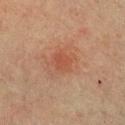{"biopsy_status": "not biopsied; imaged during a skin examination", "patient": {"sex": "female", "age_approx": 55}, "lighting": "cross-polarized", "image": {"source": "total-body photography crop", "field_of_view_mm": 15}, "automated_metrics": {"cielab_L": 40, "cielab_a": 22, "cielab_b": 27, "vs_skin_darker_L": 5.0, "vs_skin_contrast_norm": 5.0, "border_irregularity_0_10": 2.0, "nevus_likeness_0_100": 5, "lesion_detection_confidence_0_100": 100}, "site": "chest"}